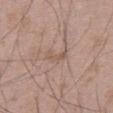notes = total-body-photography surveillance lesion; no biopsy | tile lighting = white-light illumination | image = ~15 mm crop, total-body skin-cancer survey | patient = male, aged approximately 50 | size = ~3 mm (longest diameter) | anatomic site = the abdomen.